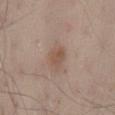This is a white-light tile. Located on the mid back. A roughly 15 mm field-of-view crop from a total-body skin photograph. Measured at roughly 3 mm in maximum diameter. A male patient, aged around 55.